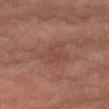notes = no biopsy performed (imaged during a skin exam)
image source = ~15 mm tile from a whole-body skin photo
patient = male, in their mid-70s
anatomic site = the left thigh
lighting = cross-polarized
lesion size = about 4 mm